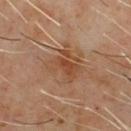Impression: The lesion was tiled from a total-body skin photograph and was not biopsied. Clinical summary: A close-up tile cropped from a whole-body skin photograph, about 15 mm across. The total-body-photography lesion software estimated a lesion area of about 15 mm² and two-axis asymmetry of about 0.25. It also reported roughly 7 lightness units darker than nearby skin. The subject is a male aged approximately 60. From the chest.